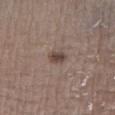A male subject aged 73 to 77. A close-up tile cropped from a whole-body skin photograph, about 15 mm across. The total-body-photography lesion software estimated a lesion area of about 3.5 mm², a shape eccentricity near 0.75, and a shape-asymmetry score of about 0.2 (0 = symmetric). Located on the left lower leg.